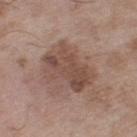| key | value |
|---|---|
| biopsy status | no biopsy performed (imaged during a skin exam) |
| acquisition | ~15 mm crop, total-body skin-cancer survey |
| patient | male, roughly 50 years of age |
| tile lighting | white-light |
| automated lesion analysis | a footprint of about 19 mm² and a symmetry-axis asymmetry near 0.3; a color-variation rating of about 4.5/10 and peripheral color asymmetry of about 1.5; an automated nevus-likeness rating near 0 out of 100 and a lesion-detection confidence of about 100/100 |
| location | the right thigh |
| diameter | about 5.5 mm |